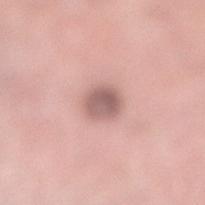Assessment: This lesion was catalogued during total-body skin photography and was not selected for biopsy. Context: The subject is a female aged approximately 40. The lesion is located on the leg. This is a white-light tile. A roughly 15 mm field-of-view crop from a total-body skin photograph.